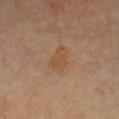<tbp_lesion>
<biopsy_status>not biopsied; imaged during a skin examination</biopsy_status>
<site>front of the torso</site>
<image>
  <source>total-body photography crop</source>
  <field_of_view_mm>15</field_of_view_mm>
</image>
<patient>
  <sex>male</sex>
  <age_approx>65</age_approx>
</patient>
</tbp_lesion>